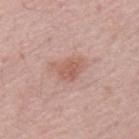Q: Was a biopsy performed?
A: imaged on a skin check; not biopsied
Q: Who is the patient?
A: male, aged 63 to 67
Q: What is the anatomic site?
A: the mid back
Q: What did automated image analysis measure?
A: a mean CIELAB color near L≈58 a*≈22 b*≈26 and a normalized lesion–skin contrast near 6; a color-variation rating of about 2/10 and a peripheral color-asymmetry measure near 0.5; a nevus-likeness score of about 40/100 and lesion-presence confidence of about 100/100
Q: What is the imaging modality?
A: ~15 mm crop, total-body skin-cancer survey